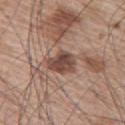Recorded during total-body skin imaging; not selected for excision or biopsy. From the upper back. Imaged with white-light lighting. Longest diameter approximately 3.5 mm. Cropped from a total-body skin-imaging series; the visible field is about 15 mm. A male patient approximately 65 years of age.